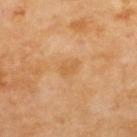Captured during whole-body skin photography for melanoma surveillance; the lesion was not biopsied. This image is a 15 mm lesion crop taken from a total-body photograph. A male patient in their 70s. Located on the upper back.